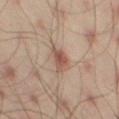Impression:
This lesion was catalogued during total-body skin photography and was not selected for biopsy.
Clinical summary:
Imaged with cross-polarized lighting. The lesion is located on the left thigh. This image is a 15 mm lesion crop taken from a total-body photograph. A male patient in their mid- to late 40s. Longest diameter approximately 3 mm.